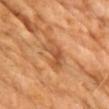Part of a total-body skin-imaging series; this lesion was reviewed on a skin check and was not flagged for biopsy. The recorded lesion diameter is about 4 mm. A 15 mm close-up tile from a total-body photography series done for melanoma screening. The subject is a male aged approximately 70. Located on the chest.